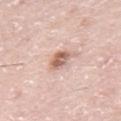  site: mid back
  automated_metrics:
    vs_skin_darker_L: 14.0
    vs_skin_contrast_norm: 8.5
    nevus_likeness_0_100: 65
    lesion_detection_confidence_0_100: 100
  lesion_size:
    long_diameter_mm_approx: 3.0
  lighting: white-light
  image:
    source: total-body photography crop
    field_of_view_mm: 15
  patient:
    sex: male
    age_approx: 75